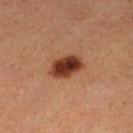This is a cross-polarized tile. Cropped from a whole-body photographic skin survey; the tile spans about 15 mm. On the left lower leg. Automated tile analysis of the lesion measured a lesion area of about 9.5 mm² and a shape eccentricity near 0.75. The software also gave an automated nevus-likeness rating near 100 out of 100 and a lesion-detection confidence of about 100/100. A female subject roughly 50 years of age. The lesion's longest dimension is about 4.5 mm.